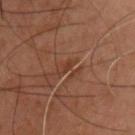Assessment: Recorded during total-body skin imaging; not selected for excision or biopsy. Image and clinical context: The subject is a male roughly 45 years of age. Automated tile analysis of the lesion measured an eccentricity of roughly 0.8 and two-axis asymmetry of about 0.55. It also reported a border-irregularity rating of about 4/10, internal color variation of about 0 on a 0–10 scale, and radial color variation of about 0. This image is a 15 mm lesion crop taken from a total-body photograph. Located on the front of the torso.